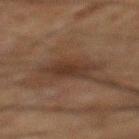Q: Was this lesion biopsied?
A: imaged on a skin check; not biopsied
Q: What kind of image is this?
A: ~15 mm crop, total-body skin-cancer survey
Q: Patient demographics?
A: male, about 65 years old
Q: Automated lesion metrics?
A: an area of roughly 10 mm² and an outline eccentricity of about 0.85 (0 = round, 1 = elongated); a mean CIELAB color near L≈27 a*≈14 b*≈21, about 7 CIELAB-L* units darker than the surrounding skin, and a lesion-to-skin contrast of about 7.5 (normalized; higher = more distinct); border irregularity of about 3 on a 0–10 scale, a color-variation rating of about 2/10, and peripheral color asymmetry of about 0.5; an automated nevus-likeness rating near 20 out of 100 and a lesion-detection confidence of about 100/100
Q: How was the tile lit?
A: cross-polarized
Q: Where on the body is the lesion?
A: the mid back
Q: Lesion size?
A: ≈5 mm